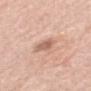Clinical impression:
The lesion was tiled from a total-body skin photograph and was not biopsied.
Acquisition and patient details:
The total-body-photography lesion software estimated a lesion–skin lightness drop of about 11 and a normalized border contrast of about 6.5. The software also gave a classifier nevus-likeness of about 15/100 and lesion-presence confidence of about 100/100. The lesion's longest dimension is about 3 mm. A male subject, aged 78 to 82. A roughly 15 mm field-of-view crop from a total-body skin photograph. Located on the mid back.